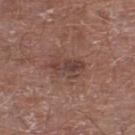| key | value |
|---|---|
| workup | imaged on a skin check; not biopsied |
| body site | the right lower leg |
| imaging modality | 15 mm crop, total-body photography |
| tile lighting | white-light |
| patient | male, roughly 70 years of age |